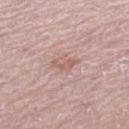Imaged during a routine full-body skin examination; the lesion was not biopsied and no histopathology is available.
About 2.5 mm across.
The patient is a female roughly 60 years of age.
A 15 mm close-up extracted from a 3D total-body photography capture.
The total-body-photography lesion software estimated an outline eccentricity of about 0.85 (0 = round, 1 = elongated). The analysis additionally found a detector confidence of about 100 out of 100 that the crop contains a lesion.
The tile uses white-light illumination.
On the left thigh.